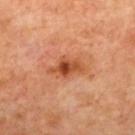<tbp_lesion>
  <biopsy_status>not biopsied; imaged during a skin examination</biopsy_status>
  <lesion_size>
    <long_diameter_mm_approx>4.5</long_diameter_mm_approx>
  </lesion_size>
  <image>
    <source>total-body photography crop</source>
    <field_of_view_mm>15</field_of_view_mm>
  </image>
  <automated_metrics>
    <cielab_L>51</cielab_L>
    <cielab_a>29</cielab_a>
    <cielab_b>39</cielab_b>
    <vs_skin_darker_L>11.0</vs_skin_darker_L>
    <vs_skin_contrast_norm>8.0</vs_skin_contrast_norm>
    <border_irregularity_0_10>3.5</border_irregularity_0_10>
    <peripheral_color_asymmetry>2.0</peripheral_color_asymmetry>
  </automated_metrics>
  <site>mid back</site>
  <lighting>cross-polarized</lighting>
  <patient>
    <age_approx>65</age_approx>
  </patient>
</tbp_lesion>